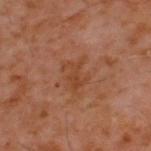{
  "site": "upper back",
  "image": {
    "source": "total-body photography crop",
    "field_of_view_mm": 15
  },
  "lesion_size": {
    "long_diameter_mm_approx": 3.5
  },
  "lighting": "cross-polarized",
  "automated_metrics": {
    "area_mm2_approx": 5.5,
    "eccentricity": 0.7,
    "shape_asymmetry": 0.45,
    "cielab_L": 40,
    "cielab_a": 23,
    "cielab_b": 32,
    "vs_skin_contrast_norm": 5.5,
    "border_irregularity_0_10": 7.5,
    "peripheral_color_asymmetry": 0.5,
    "nevus_likeness_0_100": 0,
    "lesion_detection_confidence_0_100": 100
  },
  "patient": {
    "sex": "male",
    "age_approx": 60
  }
}